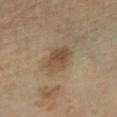notes = total-body-photography surveillance lesion; no biopsy | subject = male, aged approximately 65 | size = about 4 mm | image = ~15 mm tile from a whole-body skin photo | anatomic site = the left lower leg | TBP lesion metrics = border irregularity of about 2.5 on a 0–10 scale, a within-lesion color-variation index near 3.5/10, and a peripheral color-asymmetry measure near 1.